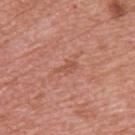Impression: This lesion was catalogued during total-body skin photography and was not selected for biopsy. Image and clinical context: From the upper back. A 15 mm close-up tile from a total-body photography series done for melanoma screening. A male patient aged 68–72.